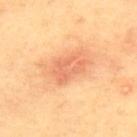<lesion>
<biopsy_status>not biopsied; imaged during a skin examination</biopsy_status>
<lighting>cross-polarized</lighting>
<site>upper back</site>
<patient>
  <sex>male</sex>
  <age_approx>55</age_approx>
</patient>
<automated_metrics>
  <border_irregularity_0_10>3.0</border_irregularity_0_10>
  <peripheral_color_asymmetry>1.0</peripheral_color_asymmetry>
  <nevus_likeness_0_100>85</nevus_likeness_0_100>
  <lesion_detection_confidence_0_100>100</lesion_detection_confidence_0_100>
</automated_metrics>
<image>
  <source>total-body photography crop</source>
  <field_of_view_mm>15</field_of_view_mm>
</image>
</lesion>